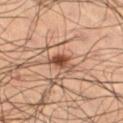The lesion was tiled from a total-body skin photograph and was not biopsied. The lesion is on the left thigh. The subject is a male aged 63–67. Longest diameter approximately 2.5 mm. Captured under cross-polarized illumination. An algorithmic analysis of the crop reported an automated nevus-likeness rating near 100 out of 100. This image is a 15 mm lesion crop taken from a total-body photograph.